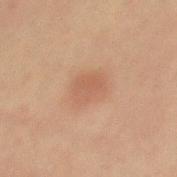notes — total-body-photography surveillance lesion; no biopsy
location — the mid back
patient — male, aged 58 to 62
image — ~15 mm tile from a whole-body skin photo